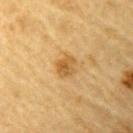Acquisition and patient details: The lesion-visualizer software estimated a border-irregularity rating of about 1.5/10, a color-variation rating of about 3.5/10, and peripheral color asymmetry of about 1. The software also gave a classifier nevus-likeness of about 70/100. This is a cross-polarized tile. The lesion is on the left upper arm. A male subject, aged 83 or over. A lesion tile, about 15 mm wide, cut from a 3D total-body photograph. Approximately 2.5 mm at its widest.